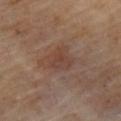Clinical impression: The lesion was tiled from a total-body skin photograph and was not biopsied. Background: Cropped from a whole-body photographic skin survey; the tile spans about 15 mm. On the left upper arm. Captured under cross-polarized illumination. An algorithmic analysis of the crop reported an average lesion color of about L≈40 a*≈18 b*≈25 (CIELAB), a lesion–skin lightness drop of about 6, and a normalized border contrast of about 5.5. And it measured an automated nevus-likeness rating near 0 out of 100 and a lesion-detection confidence of about 100/100. A male subject, approximately 70 years of age.